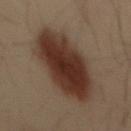{"biopsy_status": "not biopsied; imaged during a skin examination"}